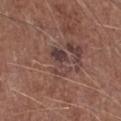follow-up: imaged on a skin check; not biopsied | subject: male, in their mid-70s | acquisition: ~15 mm crop, total-body skin-cancer survey | site: the right lower leg.